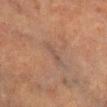Q: Was this lesion biopsied?
A: imaged on a skin check; not biopsied
Q: What is the imaging modality?
A: ~15 mm crop, total-body skin-cancer survey
Q: Lesion size?
A: ≈3 mm
Q: Who is the patient?
A: male, about 70 years old
Q: What is the anatomic site?
A: the left lower leg
Q: How was the tile lit?
A: cross-polarized